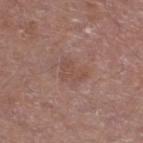Captured during whole-body skin photography for melanoma surveillance; the lesion was not biopsied. Cropped from a whole-body photographic skin survey; the tile spans about 15 mm. The total-body-photography lesion software estimated a lesion area of about 5 mm², a shape eccentricity near 0.75, and a shape-asymmetry score of about 0.5 (0 = symmetric). The software also gave about 6 CIELAB-L* units darker than the surrounding skin and a normalized border contrast of about 4.5. And it measured a color-variation rating of about 1.5/10 and peripheral color asymmetry of about 0.5. The patient is a male aged 68–72. The lesion is on the left lower leg. The lesion's longest dimension is about 3 mm.